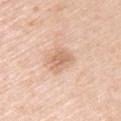follow-up=catalogued during a skin exam; not biopsied
patient=female, aged approximately 65
acquisition=total-body-photography crop, ~15 mm field of view
location=the back
diameter=~3 mm (longest diameter)